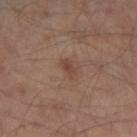Impression:
Imaged during a routine full-body skin examination; the lesion was not biopsied and no histopathology is available.
Acquisition and patient details:
Cropped from a total-body skin-imaging series; the visible field is about 15 mm. The lesion is located on the left thigh. The patient is a male roughly 65 years of age.